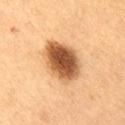biopsy status=total-body-photography surveillance lesion; no biopsy | illumination=cross-polarized illumination | acquisition=~15 mm tile from a whole-body skin photo | location=the lower back | lesion diameter=about 6 mm | subject=male, aged approximately 55.